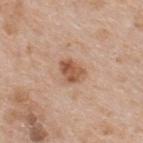The lesion-visualizer software estimated a footprint of about 6 mm², a shape eccentricity near 0.65, and two-axis asymmetry of about 0.25. It also reported an average lesion color of about L≈55 a*≈22 b*≈32 (CIELAB) and about 12 CIELAB-L* units darker than the surrounding skin.
The lesion's longest dimension is about 3.5 mm.
A roughly 15 mm field-of-view crop from a total-body skin photograph.
The lesion is on the upper back.
A male subject aged approximately 65.
This is a white-light tile.